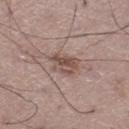Q: What did automated image analysis measure?
A: an average lesion color of about L≈50 a*≈17 b*≈23 (CIELAB) and a lesion–skin lightness drop of about 10; a border-irregularity index near 4/10 and radial color variation of about 2; a nevus-likeness score of about 10/100
Q: Patient demographics?
A: male, about 50 years old
Q: What is the anatomic site?
A: the left thigh
Q: Illumination type?
A: white-light illumination
Q: How was this image acquired?
A: ~15 mm tile from a whole-body skin photo
Q: What is the lesion's diameter?
A: ≈3.5 mm